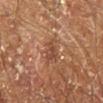biopsy_status: not biopsied; imaged during a skin examination
patient:
  sex: male
  age_approx: 60
site: right leg
lesion_size:
  long_diameter_mm_approx: 3.5
image:
  source: total-body photography crop
  field_of_view_mm: 15